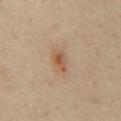| feature | finding |
|---|---|
| workup | imaged on a skin check; not biopsied |
| image source | total-body-photography crop, ~15 mm field of view |
| illumination | cross-polarized illumination |
| automated metrics | a lesion area of about 5 mm², an eccentricity of roughly 0.8, and two-axis asymmetry of about 0.2; a border-irregularity index near 2/10, internal color variation of about 5 on a 0–10 scale, and a peripheral color-asymmetry measure near 1.5; a detector confidence of about 100 out of 100 that the crop contains a lesion |
| location | the back |
| size | ≈3 mm |
| patient | male, aged 53 to 57 |